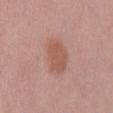notes: imaged on a skin check; not biopsied | body site: the mid back | acquisition: total-body-photography crop, ~15 mm field of view | patient: female, aged around 60.